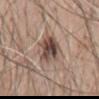Impression:
This lesion was catalogued during total-body skin photography and was not selected for biopsy.
Background:
Imaged with white-light lighting. A close-up tile cropped from a whole-body skin photograph, about 15 mm across. Located on the mid back. A male patient aged approximately 60. Approximately 4.5 mm at its widest.